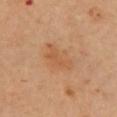Case summary:
– biopsy status · imaged on a skin check; not biopsied
– acquisition · 15 mm crop, total-body photography
– patient · male, in their mid-60s
– site · the chest
– lesion size · ≈5 mm